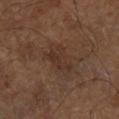Case summary:
• workup: total-body-photography surveillance lesion; no biopsy
• anatomic site: the left lower leg
• illumination: cross-polarized illumination
• patient: about 65 years old
• image source: 15 mm crop, total-body photography